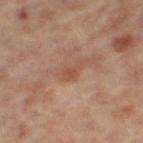Q: Was a biopsy performed?
A: total-body-photography surveillance lesion; no biopsy
Q: How was the tile lit?
A: cross-polarized
Q: Patient demographics?
A: female, approximately 65 years of age
Q: What kind of image is this?
A: 15 mm crop, total-body photography
Q: Automated lesion metrics?
A: an area of roughly 3 mm²; a normalized lesion–skin contrast near 5.5; a detector confidence of about 100 out of 100 that the crop contains a lesion
Q: Where on the body is the lesion?
A: the left leg
Q: What is the lesion's diameter?
A: ~2.5 mm (longest diameter)